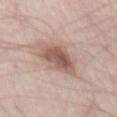{"biopsy_status": "not biopsied; imaged during a skin examination", "lighting": "white-light", "patient": {"sex": "male", "age_approx": 60}, "image": {"source": "total-body photography crop", "field_of_view_mm": 15}, "site": "abdomen", "lesion_size": {"long_diameter_mm_approx": 5.5}}